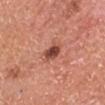Impression: The lesion was photographed on a routine skin check and not biopsied; there is no pathology result. Image and clinical context: Automated image analysis of the tile measured an automated nevus-likeness rating near 90 out of 100 and a lesion-detection confidence of about 100/100. A male patient, in their mid- to late 50s. The lesion is located on the head or neck. Imaged with white-light lighting. This image is a 15 mm lesion crop taken from a total-body photograph. Measured at roughly 2.5 mm in maximum diameter.